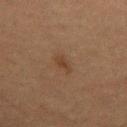Imaged during a routine full-body skin examination; the lesion was not biopsied and no histopathology is available. This is a cross-polarized tile. Longest diameter approximately 2.5 mm. On the upper back. The total-body-photography lesion software estimated a footprint of about 2.5 mm², a shape eccentricity near 0.9, and a symmetry-axis asymmetry near 0.3. It also reported a lesion color around L≈32 a*≈15 b*≈25 in CIELAB, roughly 5 lightness units darker than nearby skin, and a normalized border contrast of about 6. And it measured a nevus-likeness score of about 40/100 and a lesion-detection confidence of about 100/100. A roughly 15 mm field-of-view crop from a total-body skin photograph. A female patient, aged around 60.